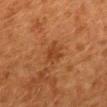Findings:
– follow-up — imaged on a skin check; not biopsied
– imaging modality — 15 mm crop, total-body photography
– location — the mid back
– image-analysis metrics — a lesion area of about 4 mm² and a shape-asymmetry score of about 0.3 (0 = symmetric); a mean CIELAB color near L≈34 a*≈22 b*≈34 and a lesion-to-skin contrast of about 6 (normalized; higher = more distinct); a within-lesion color-variation index near 1.5/10 and peripheral color asymmetry of about 0.5; lesion-presence confidence of about 100/100
– lesion size — about 2.5 mm
– illumination — cross-polarized
– subject — female, aged 48–52